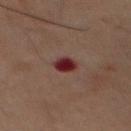biopsy_status: not biopsied; imaged during a skin examination
site: chest
lighting: cross-polarized
image:
  source: total-body photography crop
  field_of_view_mm: 15
automated_metrics:
  cielab_L: 21
  cielab_a: 22
  cielab_b: 16
  vs_skin_darker_L: 11.0
  vs_skin_contrast_norm: 13.0
patient:
  sex: male
  age_approx: 60
lesion_size:
  long_diameter_mm_approx: 2.5